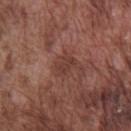No biopsy was performed on this lesion — it was imaged during a full skin examination and was not determined to be concerning. The recorded lesion diameter is about 3 mm. The tile uses white-light illumination. On the front of the torso. Cropped from a total-body skin-imaging series; the visible field is about 15 mm. The patient is a male in their mid- to late 70s.